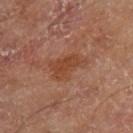Part of a total-body skin-imaging series; this lesion was reviewed on a skin check and was not flagged for biopsy. A region of skin cropped from a whole-body photographic capture, roughly 15 mm wide. On the right thigh. Measured at roughly 4.5 mm in maximum diameter. The patient is a male aged 68–72.